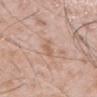Recorded during total-body skin imaging; not selected for excision or biopsy. From the mid back. The lesion's longest dimension is about 3 mm. A male subject aged 48 to 52. The lesion-visualizer software estimated an outline eccentricity of about 0.95 (0 = round, 1 = elongated) and two-axis asymmetry of about 0.45. It also reported a lesion-to-skin contrast of about 6 (normalized; higher = more distinct). And it measured a nevus-likeness score of about 0/100 and lesion-presence confidence of about 100/100. Cropped from a total-body skin-imaging series; the visible field is about 15 mm.